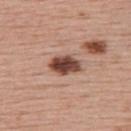Recorded during total-body skin imaging; not selected for excision or biopsy. The tile uses white-light illumination. Automated tile analysis of the lesion measured a lesion area of about 8 mm², an outline eccentricity of about 0.8 (0 = round, 1 = elongated), and a symmetry-axis asymmetry near 0.15. The software also gave an average lesion color of about L≈46 a*≈22 b*≈26 (CIELAB), roughly 18 lightness units darker than nearby skin, and a normalized border contrast of about 12.5. It also reported border irregularity of about 1.5 on a 0–10 scale and a peripheral color-asymmetry measure near 2. The analysis additionally found a classifier nevus-likeness of about 100/100 and a lesion-detection confidence of about 100/100. The recorded lesion diameter is about 4 mm. A female subject, aged 53–57. A lesion tile, about 15 mm wide, cut from a 3D total-body photograph. Located on the upper back.